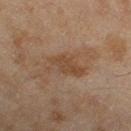Cropped from a whole-body photographic skin survey; the tile spans about 15 mm. A female patient, roughly 60 years of age. Imaged with cross-polarized lighting. The total-body-photography lesion software estimated an outline eccentricity of about 0.85 (0 = round, 1 = elongated) and a shape-asymmetry score of about 0.35 (0 = symmetric). The analysis additionally found a lesion color around L≈41 a*≈16 b*≈28 in CIELAB, about 7 CIELAB-L* units darker than the surrounding skin, and a normalized border contrast of about 6. It also reported a nevus-likeness score of about 0/100. Approximately 4.5 mm at its widest. On the right thigh.